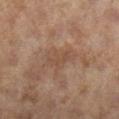Notes:
• follow-up: imaged on a skin check; not biopsied
• subject: female, about 60 years old
• illumination: cross-polarized illumination
• acquisition: ~15 mm crop, total-body skin-cancer survey
• body site: the right lower leg
• size: about 3.5 mm